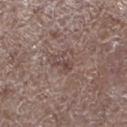Q: Was this lesion biopsied?
A: catalogued during a skin exam; not biopsied